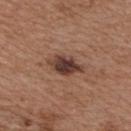<case>
  <biopsy_status>not biopsied; imaged during a skin examination</biopsy_status>
  <patient>
    <sex>male</sex>
    <age_approx>55</age_approx>
  </patient>
  <lesion_size>
    <long_diameter_mm_approx>4.5</long_diameter_mm_approx>
  </lesion_size>
  <image>
    <source>total-body photography crop</source>
    <field_of_view_mm>15</field_of_view_mm>
  </image>
  <automated_metrics>
    <area_mm2_approx>7.5</area_mm2_approx>
    <eccentricity>0.85</eccentricity>
    <cielab_L>39</cielab_L>
    <cielab_a>19</cielab_a>
    <cielab_b>24</cielab_b>
    <vs_skin_darker_L>14.0</vs_skin_darker_L>
    <vs_skin_contrast_norm>11.5</vs_skin_contrast_norm>
    <border_irregularity_0_10>3.0</border_irregularity_0_10>
    <color_variation_0_10>4.0</color_variation_0_10>
    <peripheral_color_asymmetry>1.0</peripheral_color_asymmetry>
  </automated_metrics>
  <site>chest</site>
  <lighting>white-light</lighting>
</case>